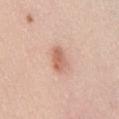The lesion was photographed on a routine skin check and not biopsied; there is no pathology result. The recorded lesion diameter is about 3.5 mm. A 15 mm close-up extracted from a 3D total-body photography capture. A male patient, aged 33–37. Located on the front of the torso.